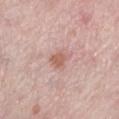{
  "biopsy_status": "not biopsied; imaged during a skin examination",
  "automated_metrics": {
    "eccentricity": 0.55,
    "shape_asymmetry": 0.35,
    "cielab_L": 60,
    "cielab_a": 22,
    "cielab_b": 26,
    "vs_skin_darker_L": 9.0,
    "vs_skin_contrast_norm": 7.0,
    "border_irregularity_0_10": 3.0,
    "color_variation_0_10": 1.5,
    "peripheral_color_asymmetry": 0.5,
    "nevus_likeness_0_100": 35
  },
  "lighting": "white-light",
  "site": "left lower leg",
  "patient": {
    "sex": "female",
    "age_approx": 45
  },
  "image": {
    "source": "total-body photography crop",
    "field_of_view_mm": 15
  }
}